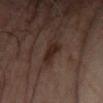biopsy_status: not biopsied; imaged during a skin examination
patient:
  sex: male
  age_approx: 65
image:
  source: total-body photography crop
  field_of_view_mm: 15
lighting: cross-polarized
site: left forearm